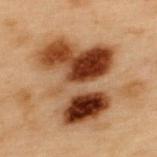{"biopsy_status": "not biopsied; imaged during a skin examination", "site": "upper back", "image": {"source": "total-body photography crop", "field_of_view_mm": 15}, "lighting": "cross-polarized", "patient": {"sex": "male", "age_approx": 55}, "lesion_size": {"long_diameter_mm_approx": 9.0}}